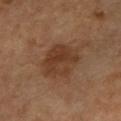Part of a total-body skin-imaging series; this lesion was reviewed on a skin check and was not flagged for biopsy. Longest diameter approximately 4.5 mm. On the left arm. Automated tile analysis of the lesion measured a border-irregularity rating of about 2/10, internal color variation of about 4 on a 0–10 scale, and a peripheral color-asymmetry measure near 1.5. This is a cross-polarized tile. A female subject, aged 58–62. Cropped from a whole-body photographic skin survey; the tile spans about 15 mm.